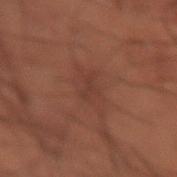The lesion was photographed on a routine skin check and not biopsied; there is no pathology result. Located on the back. Approximately 3 mm at its widest. A male subject in their 50s. A 15 mm close-up extracted from a 3D total-body photography capture. This is a cross-polarized tile.